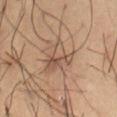{
  "patient": {
    "sex": "male",
    "age_approx": 55
  },
  "site": "abdomen",
  "image": {
    "source": "total-body photography crop",
    "field_of_view_mm": 15
  }
}